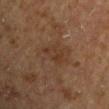<lesion>
  <biopsy_status>not biopsied; imaged during a skin examination</biopsy_status>
  <lighting>cross-polarized</lighting>
  <automated_metrics>
    <area_mm2_approx>5.0</area_mm2_approx>
    <eccentricity>0.8</eccentricity>
    <shape_asymmetry>0.35</shape_asymmetry>
    <cielab_L>31</cielab_L>
    <cielab_a>16</cielab_a>
    <cielab_b>26</cielab_b>
    <vs_skin_darker_L>5.0</vs_skin_darker_L>
    <vs_skin_contrast_norm>5.0</vs_skin_contrast_norm>
    <border_irregularity_0_10>4.0</border_irregularity_0_10>
    <color_variation_0_10>2.5</color_variation_0_10>
    <peripheral_color_asymmetry>0.5</peripheral_color_asymmetry>
    <nevus_likeness_0_100>0</nevus_likeness_0_100>
    <lesion_detection_confidence_0_100>100</lesion_detection_confidence_0_100>
  </automated_metrics>
  <lesion_size>
    <long_diameter_mm_approx>3.5</long_diameter_mm_approx>
  </lesion_size>
  <patient>
    <sex>male</sex>
    <age_approx>65</age_approx>
  </patient>
  <site>arm</site>
  <image>
    <source>total-body photography crop</source>
    <field_of_view_mm>15</field_of_view_mm>
  </image>
</lesion>